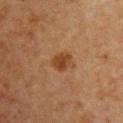Recorded during total-body skin imaging; not selected for excision or biopsy.
The subject is a female aged around 60.
From the chest.
A lesion tile, about 15 mm wide, cut from a 3D total-body photograph.
This is a cross-polarized tile.